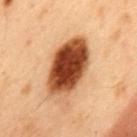{
  "biopsy_status": "not biopsied; imaged during a skin examination",
  "image": {
    "source": "total-body photography crop",
    "field_of_view_mm": 15
  },
  "lesion_size": {
    "long_diameter_mm_approx": 7.5
  },
  "lighting": "cross-polarized",
  "patient": {
    "sex": "male",
    "age_approx": 55
  },
  "site": "mid back"
}